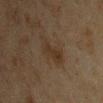About 4.5 mm across. Located on the arm. A roughly 15 mm field-of-view crop from a total-body skin photograph. Imaged with cross-polarized lighting. The subject is a male aged 43–47.